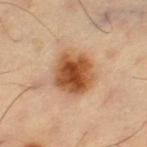Q: Was a biopsy performed?
A: total-body-photography surveillance lesion; no biopsy
Q: Where on the body is the lesion?
A: the left thigh
Q: What are the patient's age and sex?
A: male, aged 58 to 62
Q: How was the tile lit?
A: cross-polarized illumination
Q: Automated lesion metrics?
A: a classifier nevus-likeness of about 100/100
Q: Lesion size?
A: ≈4.5 mm
Q: What is the imaging modality?
A: total-body-photography crop, ~15 mm field of view